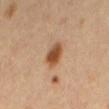| key | value |
|---|---|
| notes | catalogued during a skin exam; not biopsied |
| subject | female, aged around 35 |
| lighting | cross-polarized illumination |
| image source | ~15 mm crop, total-body skin-cancer survey |
| size | about 3.5 mm |
| anatomic site | the mid back |